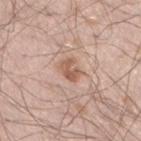This lesion was catalogued during total-body skin photography and was not selected for biopsy. Imaged with white-light lighting. Approximately 3 mm at its widest. A region of skin cropped from a whole-body photographic capture, roughly 15 mm wide. On the left lower leg. Automated image analysis of the tile measured a footprint of about 4.5 mm², an eccentricity of roughly 0.7, and a symmetry-axis asymmetry near 0.25. And it measured a classifier nevus-likeness of about 60/100. The patient is a male in their mid- to late 20s.